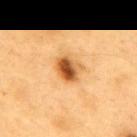Q: What is the imaging modality?
A: 15 mm crop, total-body photography
Q: How was the tile lit?
A: cross-polarized
Q: Lesion size?
A: about 3.5 mm
Q: Lesion location?
A: the mid back
Q: Who is the patient?
A: male, in their 60s